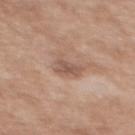Q: Was a biopsy performed?
A: imaged on a skin check; not biopsied
Q: What kind of image is this?
A: 15 mm crop, total-body photography
Q: What is the anatomic site?
A: the mid back
Q: Patient demographics?
A: female, about 40 years old
Q: What lighting was used for the tile?
A: white-light illumination